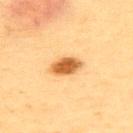<case>
  <biopsy_status>not biopsied; imaged during a skin examination</biopsy_status>
  <patient>
    <sex>female</sex>
    <age_approx>55</age_approx>
  </patient>
  <lesion_size>
    <long_diameter_mm_approx>3.5</long_diameter_mm_approx>
  </lesion_size>
  <lighting>cross-polarized</lighting>
  <site>upper back</site>
  <image>
    <source>total-body photography crop</source>
    <field_of_view_mm>15</field_of_view_mm>
  </image>
</case>